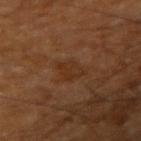* workup — imaged on a skin check; not biopsied
* image — 15 mm crop, total-body photography
* subject — male, roughly 60 years of age
* site — the right upper arm
* lighting — cross-polarized illumination